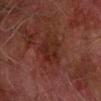Q: Was a biopsy performed?
A: catalogued during a skin exam; not biopsied
Q: Automated lesion metrics?
A: a border-irregularity rating of about 3/10, internal color variation of about 3.5 on a 0–10 scale, and peripheral color asymmetry of about 1; an automated nevus-likeness rating near 5 out of 100
Q: What is the imaging modality?
A: total-body-photography crop, ~15 mm field of view
Q: What is the lesion's diameter?
A: about 4 mm
Q: Patient demographics?
A: male, aged around 75
Q: How was the tile lit?
A: cross-polarized illumination
Q: What is the anatomic site?
A: the right forearm